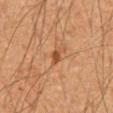follow-up — catalogued during a skin exam; not biopsied
automated lesion analysis — an average lesion color of about L≈45 a*≈23 b*≈35 (CIELAB), a lesion–skin lightness drop of about 9, and a normalized border contrast of about 7.5; a nevus-likeness score of about 35/100 and a detector confidence of about 100 out of 100 that the crop contains a lesion
image source — total-body-photography crop, ~15 mm field of view
lighting — cross-polarized illumination
anatomic site — the mid back
size — ≈2.5 mm
patient — male, aged 58 to 62